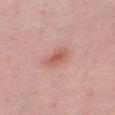Case summary:
• biopsy status — catalogued during a skin exam; not biopsied
• location — the right thigh
• size — ≈3 mm
• image — 15 mm crop, total-body photography
• lighting — white-light
• TBP lesion metrics — an average lesion color of about L≈58 a*≈26 b*≈27 (CIELAB), roughly 10 lightness units darker than nearby skin, and a lesion-to-skin contrast of about 6.5 (normalized; higher = more distinct); a border-irregularity rating of about 2/10 and internal color variation of about 2.5 on a 0–10 scale
• patient — female, aged 43 to 47